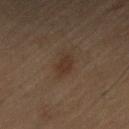Recorded during total-body skin imaging; not selected for excision or biopsy.
Imaged with cross-polarized lighting.
The lesion is located on the chest.
A male patient, in their 70s.
Measured at roughly 3.5 mm in maximum diameter.
A close-up tile cropped from a whole-body skin photograph, about 15 mm across.